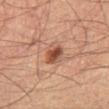About 3 mm across.
A male patient roughly 60 years of age.
A 15 mm close-up extracted from a 3D total-body photography capture.
The lesion-visualizer software estimated an area of roughly 5.5 mm² and an outline eccentricity of about 0.75 (0 = round, 1 = elongated). The software also gave a border-irregularity index near 2/10, a color-variation rating of about 5.5/10, and radial color variation of about 1.5. The software also gave a nevus-likeness score of about 90/100 and a detector confidence of about 100 out of 100 that the crop contains a lesion.